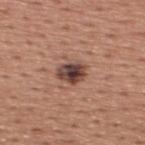The lesion was photographed on a routine skin check and not biopsied; there is no pathology result. Captured under white-light illumination. A male subject, aged approximately 45. A 15 mm crop from a total-body photograph taken for skin-cancer surveillance. Longest diameter approximately 3.5 mm. On the upper back.